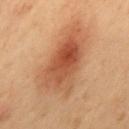Clinical impression:
Captured during whole-body skin photography for melanoma surveillance; the lesion was not biopsied.
Background:
The lesion's longest dimension is about 7 mm. From the mid back. Cropped from a total-body skin-imaging series; the visible field is about 15 mm. A male patient aged 53 to 57. This is a cross-polarized tile.